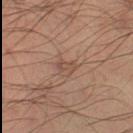biopsy status = total-body-photography surveillance lesion; no biopsy
image = total-body-photography crop, ~15 mm field of view
lesion size = about 2.5 mm
body site = the left thigh
subject = male, approximately 35 years of age